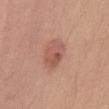{
  "biopsy_status": "not biopsied; imaged during a skin examination",
  "image": {
    "source": "total-body photography crop",
    "field_of_view_mm": 15
  },
  "patient": {
    "sex": "male",
    "age_approx": 35
  },
  "lesion_size": {
    "long_diameter_mm_approx": 3.5
  },
  "site": "abdomen",
  "automated_metrics": {
    "area_mm2_approx": 8.5,
    "eccentricity": 0.7,
    "shape_asymmetry": 0.15,
    "vs_skin_darker_L": 9.0,
    "vs_skin_contrast_norm": 6.0,
    "border_irregularity_0_10": 1.5,
    "color_variation_0_10": 5.5,
    "peripheral_color_asymmetry": 2.0,
    "lesion_detection_confidence_0_100": 100
  }
}